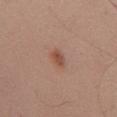Imaged during a routine full-body skin examination; the lesion was not biopsied and no histopathology is available. Cropped from a total-body skin-imaging series; the visible field is about 15 mm. The subject is a male in their 60s. On the chest.